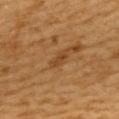Recorded during total-body skin imaging; not selected for excision or biopsy. About 3 mm across. Captured under cross-polarized illumination. A close-up tile cropped from a whole-body skin photograph, about 15 mm across. The patient is a female aged approximately 55. Located on the upper back. An algorithmic analysis of the crop reported a mean CIELAB color near L≈45 a*≈22 b*≈40, about 7 CIELAB-L* units darker than the surrounding skin, and a normalized lesion–skin contrast near 6.